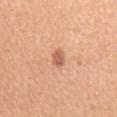site: front of the torso
image:
  source: total-body photography crop
  field_of_view_mm: 15
lighting: white-light
lesion_size:
  long_diameter_mm_approx: 2.5
patient:
  sex: male
  age_approx: 55